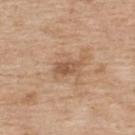Part of a total-body skin-imaging series; this lesion was reviewed on a skin check and was not flagged for biopsy. Automated tile analysis of the lesion measured a lesion area of about 4.5 mm² and an eccentricity of roughly 0.9. It also reported a border-irregularity index near 3.5/10. It also reported an automated nevus-likeness rating near 0 out of 100 and a lesion-detection confidence of about 100/100. About 3.5 mm across. On the upper back. A female subject aged around 65. Imaged with white-light lighting. A roughly 15 mm field-of-view crop from a total-body skin photograph.